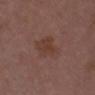notes = catalogued during a skin exam; not biopsied | image source = 15 mm crop, total-body photography | automated metrics = a lesion area of about 9.5 mm², a shape eccentricity near 0.4, and a symmetry-axis asymmetry near 0.3; a border-irregularity index near 3/10, a color-variation rating of about 2/10, and peripheral color asymmetry of about 0.5; an automated nevus-likeness rating near 5 out of 100 and a lesion-detection confidence of about 100/100 | diameter = ~3.5 mm (longest diameter) | anatomic site = the chest | patient = female, about 30 years old.